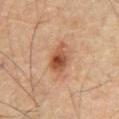Impression:
This lesion was catalogued during total-body skin photography and was not selected for biopsy.
Image and clinical context:
The lesion's longest dimension is about 4 mm. The subject is a male aged approximately 65. The tile uses cross-polarized illumination. This image is a 15 mm lesion crop taken from a total-body photograph. The lesion is on the abdomen.